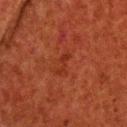Assessment: The lesion was tiled from a total-body skin photograph and was not biopsied. Clinical summary: A male subject approximately 60 years of age. From the head or neck. Captured under cross-polarized illumination. Approximately 3 mm at its widest. Cropped from a whole-body photographic skin survey; the tile spans about 15 mm.